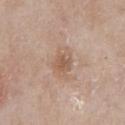This lesion was catalogued during total-body skin photography and was not selected for biopsy. Longest diameter approximately 3.5 mm. On the front of the torso. A male subject aged 63–67. A lesion tile, about 15 mm wide, cut from a 3D total-body photograph.